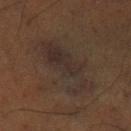This is a cross-polarized tile. A 15 mm close-up tile from a total-body photography series done for melanoma screening. The lesion is on the left lower leg. Longest diameter approximately 7.5 mm. A male patient, aged around 50. The total-body-photography lesion software estimated a shape eccentricity near 0.9. The software also gave a border-irregularity index near 6.5/10 and internal color variation of about 2.5 on a 0–10 scale.